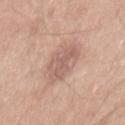Q: Is there a histopathology result?
A: imaged on a skin check; not biopsied
Q: What is the anatomic site?
A: the mid back
Q: Who is the patient?
A: male, aged approximately 30
Q: What kind of image is this?
A: 15 mm crop, total-body photography
Q: How large is the lesion?
A: ≈5 mm